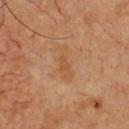| key | value |
|---|---|
| workup | no biopsy performed (imaged during a skin exam) |
| patient | male, roughly 50 years of age |
| image | total-body-photography crop, ~15 mm field of view |
| illumination | cross-polarized |
| anatomic site | the chest |
| lesion diameter | about 4 mm |